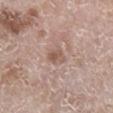notes = catalogued during a skin exam; not biopsied
patient = female, in their mid- to late 70s
automated metrics = a lesion area of about 3.5 mm², a shape eccentricity near 0.8, and two-axis asymmetry of about 0.25; a classifier nevus-likeness of about 0/100
body site = the right lower leg
tile lighting = white-light illumination
image = ~15 mm tile from a whole-body skin photo
size = ≈2.5 mm